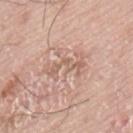| key | value |
|---|---|
| automated metrics | a nevus-likeness score of about 0/100 |
| image source | ~15 mm crop, total-body skin-cancer survey |
| patient | male, aged around 75 |
| anatomic site | the back |
| lighting | white-light |